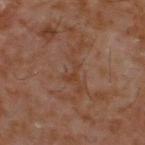The lesion was photographed on a routine skin check and not biopsied; there is no pathology result. Located on the upper back. A male patient, roughly 60 years of age. A 15 mm crop from a total-body photograph taken for skin-cancer surveillance. The lesion-visualizer software estimated an automated nevus-likeness rating near 0 out of 100 and a lesion-detection confidence of about 100/100. Measured at roughly 2.5 mm in maximum diameter.